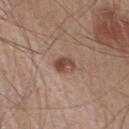No biopsy was performed on this lesion — it was imaged during a full skin examination and was not determined to be concerning. This is a white-light tile. A 15 mm close-up extracted from a 3D total-body photography capture. Located on the right lower leg. The patient is a male aged 43–47.